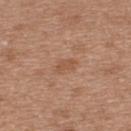Image and clinical context:
The lesion is located on the back. About 2.5 mm across. A female subject aged around 40. A region of skin cropped from a whole-body photographic capture, roughly 15 mm wide. This is a white-light tile.